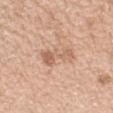follow-up: total-body-photography surveillance lesion; no biopsy